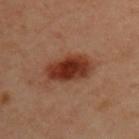| feature | finding |
|---|---|
| subject | male, approximately 50 years of age |
| site | the back |
| automated lesion analysis | a footprint of about 13 mm², a shape eccentricity near 0.85, and two-axis asymmetry of about 0.15; an average lesion color of about L≈34 a*≈26 b*≈30 (CIELAB) and a lesion-to-skin contrast of about 12 (normalized; higher = more distinct); a border-irregularity index near 2/10, internal color variation of about 4.5 on a 0–10 scale, and a peripheral color-asymmetry measure near 1; a classifier nevus-likeness of about 100/100 and lesion-presence confidence of about 100/100 |
| image | 15 mm crop, total-body photography |
| illumination | cross-polarized illumination |
| diameter | about 5.5 mm |